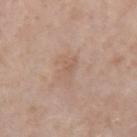Clinical impression: No biopsy was performed on this lesion — it was imaged during a full skin examination and was not determined to be concerning. Context: A female subject aged around 50. Measured at roughly 1.5 mm in maximum diameter. Captured under white-light illumination. The lesion is located on the arm. An algorithmic analysis of the crop reported an area of roughly 1.5 mm² and two-axis asymmetry of about 0.2. And it measured a color-variation rating of about 0/10 and a peripheral color-asymmetry measure near 0. It also reported a nevus-likeness score of about 0/100. A roughly 15 mm field-of-view crop from a total-body skin photograph.